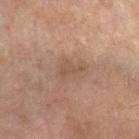Clinical impression: This lesion was catalogued during total-body skin photography and was not selected for biopsy. Clinical summary: Automated image analysis of the tile measured a lesion area of about 3 mm², an eccentricity of roughly 0.85, and two-axis asymmetry of about 0.55. It also reported an average lesion color of about L≈50 a*≈17 b*≈27 (CIELAB). Cropped from a total-body skin-imaging series; the visible field is about 15 mm. From the arm. Approximately 3 mm at its widest. Imaged with cross-polarized lighting. The subject is a female aged approximately 65.